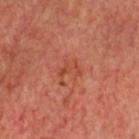{"biopsy_status": "not biopsied; imaged during a skin examination", "patient": {"sex": "male", "age_approx": 60}, "image": {"source": "total-body photography crop", "field_of_view_mm": 15}, "lighting": "cross-polarized", "site": "head or neck", "lesion_size": {"long_diameter_mm_approx": 2.5}}